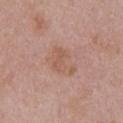follow-up — total-body-photography surveillance lesion; no biopsy
patient — female, aged approximately 30
image source — ~15 mm tile from a whole-body skin photo
location — the upper back
lesion size — about 4 mm
tile lighting — white-light illumination